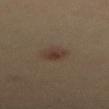Q: Was this lesion biopsied?
A: total-body-photography surveillance lesion; no biopsy
Q: What are the patient's age and sex?
A: male, roughly 40 years of age
Q: Where on the body is the lesion?
A: the abdomen
Q: How was this image acquired?
A: ~15 mm crop, total-body skin-cancer survey
Q: How was the tile lit?
A: cross-polarized
Q: What is the lesion's diameter?
A: ≈3 mm